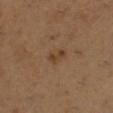{
  "biopsy_status": "not biopsied; imaged during a skin examination",
  "automated_metrics": {
    "border_irregularity_0_10": 3.5,
    "peripheral_color_asymmetry": 0.5
  },
  "site": "right lower leg",
  "patient": {
    "sex": "female",
    "age_approx": 55
  },
  "image": {
    "source": "total-body photography crop",
    "field_of_view_mm": 15
  },
  "lighting": "cross-polarized"
}